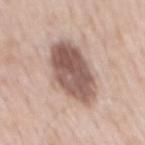{"biopsy_status": "not biopsied; imaged during a skin examination", "site": "mid back", "lesion_size": {"long_diameter_mm_approx": 7.5}, "image": {"source": "total-body photography crop", "field_of_view_mm": 15}, "automated_metrics": {"area_mm2_approx": 23.0, "eccentricity": 0.85, "shape_asymmetry": 0.15, "cielab_L": 55, "cielab_a": 17, "cielab_b": 23, "vs_skin_darker_L": 16.0, "vs_skin_contrast_norm": 10.5}, "patient": {"sex": "male", "age_approx": 50}}